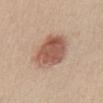Assessment: Recorded during total-body skin imaging; not selected for excision or biopsy. Context: The lesion-visualizer software estimated a shape-asymmetry score of about 0.2 (0 = symmetric). The analysis additionally found an average lesion color of about L≈55 a*≈22 b*≈29 (CIELAB) and a normalized border contrast of about 9. It also reported a border-irregularity rating of about 2/10 and internal color variation of about 4.5 on a 0–10 scale. The lesion is located on the abdomen. Measured at roughly 4.5 mm in maximum diameter. The patient is a female aged approximately 40. A lesion tile, about 15 mm wide, cut from a 3D total-body photograph. Captured under white-light illumination.